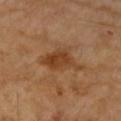workup=no biopsy performed (imaged during a skin exam) | diameter=about 5.5 mm | subject=female, about 70 years old | image source=~15 mm crop, total-body skin-cancer survey | body site=the right forearm | tile lighting=cross-polarized | image-analysis metrics=an average lesion color of about L≈43 a*≈22 b*≈36 (CIELAB), a lesion–skin lightness drop of about 9, and a normalized lesion–skin contrast near 8; internal color variation of about 3 on a 0–10 scale and radial color variation of about 1.